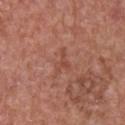Impression: Imaged during a routine full-body skin examination; the lesion was not biopsied and no histopathology is available. Clinical summary: A male subject aged 63–67. The lesion-visualizer software estimated an average lesion color of about L≈48 a*≈25 b*≈29 (CIELAB), about 6 CIELAB-L* units darker than the surrounding skin, and a lesion-to-skin contrast of about 5 (normalized; higher = more distinct). It also reported a border-irregularity rating of about 6.5/10 and a within-lesion color-variation index near 0.5/10. On the chest. Longest diameter approximately 3 mm. A 15 mm close-up extracted from a 3D total-body photography capture.